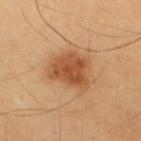This lesion was catalogued during total-body skin photography and was not selected for biopsy.
On the upper back.
A male subject aged around 35.
Captured under cross-polarized illumination.
Longest diameter approximately 5 mm.
A 15 mm crop from a total-body photograph taken for skin-cancer surveillance.
The total-body-photography lesion software estimated border irregularity of about 3 on a 0–10 scale, a color-variation rating of about 3.5/10, and peripheral color asymmetry of about 1.5.